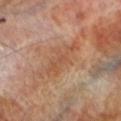Imaged during a routine full-body skin examination; the lesion was not biopsied and no histopathology is available.
A 15 mm close-up tile from a total-body photography series done for melanoma screening.
This is a cross-polarized tile.
A male subject, about 70 years old.
The lesion-visualizer software estimated a border-irregularity rating of about 6/10, a color-variation rating of about 2.5/10, and peripheral color asymmetry of about 1. And it measured an automated nevus-likeness rating near 0 out of 100 and a detector confidence of about 100 out of 100 that the crop contains a lesion.
Measured at roughly 5 mm in maximum diameter.
The lesion is on the left lower leg.